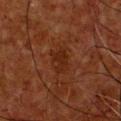The lesion was tiled from a total-body skin photograph and was not biopsied.
Cropped from a whole-body photographic skin survey; the tile spans about 15 mm.
The lesion is on the chest.
A male patient, aged 63–67.